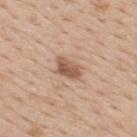  biopsy_status: not biopsied; imaged during a skin examination
  patient:
    sex: male
    age_approx: 60
  image:
    source: total-body photography crop
    field_of_view_mm: 15
  lesion_size:
    long_diameter_mm_approx: 3.0
  site: upper back